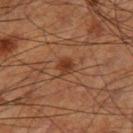  biopsy_status: not biopsied; imaged during a skin examination
  site: right thigh
  patient:
    sex: male
    age_approx: 60
  image:
    source: total-body photography crop
    field_of_view_mm: 15